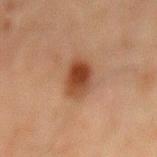notes: catalogued during a skin exam; not biopsied
imaging modality: total-body-photography crop, ~15 mm field of view
location: the back
subject: male, about 65 years old
automated lesion analysis: a footprint of about 8.5 mm², an outline eccentricity of about 0.8 (0 = round, 1 = elongated), and two-axis asymmetry of about 0.15; a peripheral color-asymmetry measure near 1.5